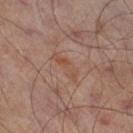* follow-up · catalogued during a skin exam; not biopsied
* imaging modality · ~15 mm crop, total-body skin-cancer survey
* location · the right thigh
* patient · male, approximately 65 years of age